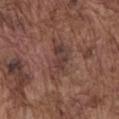follow-up: imaged on a skin check; not biopsied | body site: the chest | image source: ~15 mm crop, total-body skin-cancer survey | tile lighting: white-light illumination | subject: male, about 75 years old.